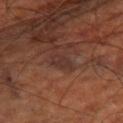This image is a 15 mm lesion crop taken from a total-body photograph.
From the right thigh.
Approximately 2.5 mm at its widest.
A patient in their mid-60s.
Imaged with cross-polarized lighting.